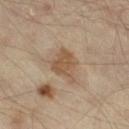This lesion was catalogued during total-body skin photography and was not selected for biopsy.
The lesion is on the right thigh.
The subject is aged 53 to 57.
The lesion's longest dimension is about 4 mm.
A 15 mm crop from a total-body photograph taken for skin-cancer surveillance.
Automated image analysis of the tile measured a lesion area of about 7.5 mm² and an outline eccentricity of about 0.65 (0 = round, 1 = elongated). It also reported a classifier nevus-likeness of about 0/100 and a detector confidence of about 100 out of 100 that the crop contains a lesion.
Captured under cross-polarized illumination.